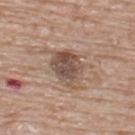{
  "biopsy_status": "not biopsied; imaged during a skin examination",
  "lesion_size": {
    "long_diameter_mm_approx": 5.0
  },
  "patient": {
    "sex": "male",
    "age_approx": 65
  },
  "automated_metrics": {
    "shape_asymmetry": 0.25,
    "cielab_L": 50,
    "cielab_a": 16,
    "cielab_b": 25,
    "vs_skin_darker_L": 12.0,
    "nevus_likeness_0_100": 45,
    "lesion_detection_confidence_0_100": 100
  },
  "site": "upper back",
  "image": {
    "source": "total-body photography crop",
    "field_of_view_mm": 15
  },
  "lighting": "white-light"
}